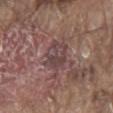The lesion was tiled from a total-body skin photograph and was not biopsied. The lesion is on the mid back. Measured at roughly 4 mm in maximum diameter. Imaged with white-light lighting. This image is a 15 mm lesion crop taken from a total-body photograph. The patient is a male in their 80s.